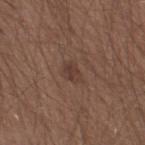{"biopsy_status": "not biopsied; imaged during a skin examination", "automated_metrics": {"border_irregularity_0_10": 2.5, "peripheral_color_asymmetry": 1.0}, "patient": {"sex": "male", "age_approx": 30}, "lesion_size": {"long_diameter_mm_approx": 2.5}, "image": {"source": "total-body photography crop", "field_of_view_mm": 15}, "site": "right thigh"}